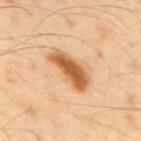Assessment: The lesion was tiled from a total-body skin photograph and was not biopsied. Background: Imaged with cross-polarized lighting. An algorithmic analysis of the crop reported a shape-asymmetry score of about 0.25 (0 = symmetric). And it measured a nevus-likeness score of about 85/100. Longest diameter approximately 5.5 mm. Cropped from a whole-body photographic skin survey; the tile spans about 15 mm. On the mid back. The subject is a male approximately 40 years of age.